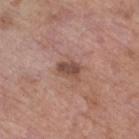A female subject aged approximately 85 or older. On the leg. About 2.5 mm across. This is a white-light tile. A 15 mm crop from a total-body photograph taken for skin-cancer surveillance.